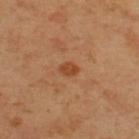Findings:
* workup · imaged on a skin check; not biopsied
* site · the upper back
* automated metrics · an eccentricity of roughly 0.75; a mean CIELAB color near L≈45 a*≈26 b*≈37 and roughly 9 lightness units darker than nearby skin; a border-irregularity rating of about 2/10, a color-variation rating of about 1/10, and a peripheral color-asymmetry measure near 0.5; a classifier nevus-likeness of about 65/100 and a lesion-detection confidence of about 100/100
* diameter · about 2.5 mm
* image · 15 mm crop, total-body photography
* patient · male, in their mid-40s